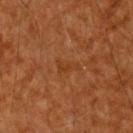Impression: Imaged during a routine full-body skin examination; the lesion was not biopsied and no histopathology is available. Image and clinical context: About 2.5 mm across. From the upper back. A male patient, in their 60s. This is a cross-polarized tile. A region of skin cropped from a whole-body photographic capture, roughly 15 mm wide.